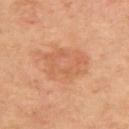Assessment: The lesion was photographed on a routine skin check and not biopsied; there is no pathology result. Clinical summary: About 6.5 mm across. Cropped from a whole-body photographic skin survey; the tile spans about 15 mm. A male subject aged 63 to 67. Automated tile analysis of the lesion measured a lesion area of about 19 mm², an outline eccentricity of about 0.75 (0 = round, 1 = elongated), and a symmetry-axis asymmetry near 0.25. And it measured a within-lesion color-variation index near 4/10. The analysis additionally found lesion-presence confidence of about 100/100. On the upper back. Imaged with cross-polarized lighting.